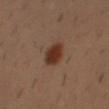Recorded during total-body skin imaging; not selected for excision or biopsy. The subject is a male about 30 years old. The lesion is on the right forearm. Approximately 3 mm at its widest. This is a cross-polarized tile. A close-up tile cropped from a whole-body skin photograph, about 15 mm across.